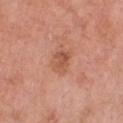Recorded during total-body skin imaging; not selected for excision or biopsy. A male subject, aged approximately 60. The lesion is located on the chest. About 3 mm across. A lesion tile, about 15 mm wide, cut from a 3D total-body photograph. Imaged with white-light lighting. An algorithmic analysis of the crop reported a lesion area of about 6.5 mm², an eccentricity of roughly 0.7, and two-axis asymmetry of about 0.15. The analysis additionally found a mean CIELAB color near L≈55 a*≈25 b*≈32, roughly 8 lightness units darker than nearby skin, and a normalized lesion–skin contrast near 6. The analysis additionally found a border-irregularity index near 1.5/10, internal color variation of about 4 on a 0–10 scale, and peripheral color asymmetry of about 1.5. It also reported an automated nevus-likeness rating near 5 out of 100 and a detector confidence of about 100 out of 100 that the crop contains a lesion.